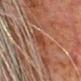Clinical impression:
Captured during whole-body skin photography for melanoma surveillance; the lesion was not biopsied.
Context:
The tile uses cross-polarized illumination. The lesion is located on the head or neck. A male patient, about 80 years old. The total-body-photography lesion software estimated a lesion–skin lightness drop of about 6 and a normalized border contrast of about 7.5. A roughly 15 mm field-of-view crop from a total-body skin photograph.